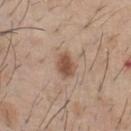{
  "automated_metrics": {
    "area_mm2_approx": 5.5,
    "border_irregularity_0_10": 1.5,
    "color_variation_0_10": 2.5,
    "peripheral_color_asymmetry": 1.0
  },
  "patient": {
    "sex": "male",
    "age_approx": 60
  },
  "lighting": "white-light",
  "lesion_size": {
    "long_diameter_mm_approx": 3.0
  },
  "image": {
    "source": "total-body photography crop",
    "field_of_view_mm": 15
  },
  "site": "chest"
}